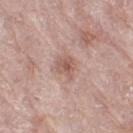Imaged with white-light lighting.
A female patient, aged around 70.
A roughly 15 mm field-of-view crop from a total-body skin photograph.
On the right thigh.
About 3 mm across.